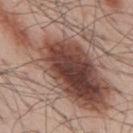| feature | finding |
|---|---|
| workup | total-body-photography surveillance lesion; no biopsy |
| illumination | white-light |
| subject | male, aged approximately 55 |
| body site | the mid back |
| image source | total-body-photography crop, ~15 mm field of view |
| diameter | ≈16.5 mm |